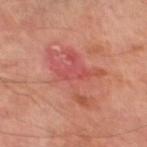follow-up: total-body-photography surveillance lesion; no biopsy
anatomic site: the left thigh
patient: male, aged 68–72
illumination: cross-polarized illumination
image: ~15 mm tile from a whole-body skin photo